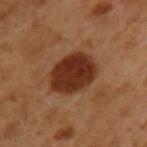– notes — catalogued during a skin exam; not biopsied
– TBP lesion metrics — a footprint of about 17 mm², an eccentricity of roughly 0.65, and a shape-asymmetry score of about 0.1 (0 = symmetric); a border-irregularity rating of about 1.5/10, a within-lesion color-variation index near 3.5/10, and a peripheral color-asymmetry measure near 1
– imaging modality — total-body-photography crop, ~15 mm field of view
– subject — male, aged approximately 50
– lesion diameter — about 5.5 mm
– site — the left upper arm
– lighting — cross-polarized illumination